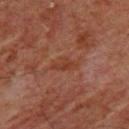Impression: The lesion was photographed on a routine skin check and not biopsied; there is no pathology result. Image and clinical context: A male subject, approximately 60 years of age. The total-body-photography lesion software estimated a nevus-likeness score of about 0/100 and a detector confidence of about 100 out of 100 that the crop contains a lesion. This image is a 15 mm lesion crop taken from a total-body photograph. From the front of the torso. About 3.5 mm across.